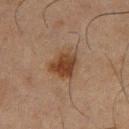– subject: male, aged around 65
– body site: the leg
– automated metrics: an area of roughly 9.5 mm², an eccentricity of roughly 0.5, and two-axis asymmetry of about 0.25
– tile lighting: cross-polarized
– imaging modality: ~15 mm tile from a whole-body skin photo